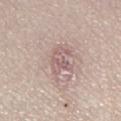The lesion was photographed on a routine skin check and not biopsied; there is no pathology result. Cropped from a whole-body photographic skin survey; the tile spans about 15 mm. About 3.5 mm across. A female patient, aged around 60. The lesion is on the left lower leg. Automated image analysis of the tile measured a lesion color around L≈59 a*≈18 b*≈19 in CIELAB, roughly 9 lightness units darker than nearby skin, and a normalized border contrast of about 6. Captured under white-light illumination.